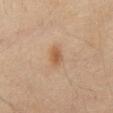No biopsy was performed on this lesion — it was imaged during a full skin examination and was not determined to be concerning.
The subject is a male in their mid- to late 60s.
Approximately 2.5 mm at its widest.
On the abdomen.
Captured under cross-polarized illumination.
A roughly 15 mm field-of-view crop from a total-body skin photograph.
Automated tile analysis of the lesion measured a lesion area of about 3.5 mm² and a shape-asymmetry score of about 0.15 (0 = symmetric). The analysis additionally found border irregularity of about 1.5 on a 0–10 scale, internal color variation of about 2.5 on a 0–10 scale, and radial color variation of about 1. The analysis additionally found a classifier nevus-likeness of about 95/100 and lesion-presence confidence of about 100/100.